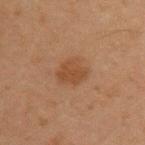notes=total-body-photography surveillance lesion; no biopsy | subject=male, in their mid-40s | size=about 3.5 mm | acquisition=15 mm crop, total-body photography | illumination=cross-polarized illumination | location=the left upper arm.